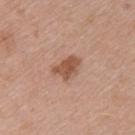Assessment:
Part of a total-body skin-imaging series; this lesion was reviewed on a skin check and was not flagged for biopsy.
Acquisition and patient details:
A female patient, aged approximately 45. The lesion is located on the left upper arm. This is a white-light tile. A 15 mm crop from a total-body photograph taken for skin-cancer surveillance. The recorded lesion diameter is about 3.5 mm.